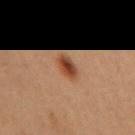Imaged during a routine full-body skin examination; the lesion was not biopsied and no histopathology is available.
The recorded lesion diameter is about 3 mm.
Automated tile analysis of the lesion measured a lesion area of about 5.5 mm², an eccentricity of roughly 0.75, and a shape-asymmetry score of about 0.15 (0 = symmetric). The analysis additionally found an average lesion color of about L≈37 a*≈19 b*≈29 (CIELAB), a lesion–skin lightness drop of about 10, and a normalized border contrast of about 9.5. The software also gave a border-irregularity rating of about 1.5/10, internal color variation of about 5 on a 0–10 scale, and a peripheral color-asymmetry measure near 1.5. The software also gave a lesion-detection confidence of about 100/100.
A female patient aged approximately 40.
On the right upper arm.
A region of skin cropped from a whole-body photographic capture, roughly 15 mm wide.